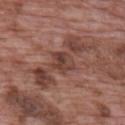Q: Is there a histopathology result?
A: no biopsy performed (imaged during a skin exam)